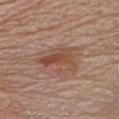Impression: The lesion was tiled from a total-body skin photograph and was not biopsied. Image and clinical context: An algorithmic analysis of the crop reported an area of roughly 14 mm², an outline eccentricity of about 0.75 (0 = round, 1 = elongated), and a symmetry-axis asymmetry near 0.35. It also reported about 8 CIELAB-L* units darker than the surrounding skin. The analysis additionally found a nevus-likeness score of about 90/100 and a detector confidence of about 100 out of 100 that the crop contains a lesion. Measured at roughly 5.5 mm in maximum diameter. The tile uses white-light illumination. The lesion is located on the chest. A male subject roughly 70 years of age. This image is a 15 mm lesion crop taken from a total-body photograph.